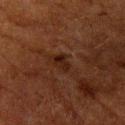patient=male, about 60 years old | imaging modality=~15 mm crop, total-body skin-cancer survey | site=the left upper arm | automated metrics=a lesion area of about 2.5 mm², a shape eccentricity near 0.85, and two-axis asymmetry of about 0.35; an automated nevus-likeness rating near 55 out of 100 and a lesion-detection confidence of about 100/100.